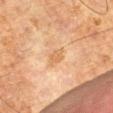Assessment: Part of a total-body skin-imaging series; this lesion was reviewed on a skin check and was not flagged for biopsy. Acquisition and patient details: Automated image analysis of the tile measured a lesion color around L≈51 a*≈17 b*≈33 in CIELAB, roughly 6 lightness units darker than nearby skin, and a lesion-to-skin contrast of about 5.5 (normalized; higher = more distinct). The analysis additionally found border irregularity of about 3 on a 0–10 scale, a color-variation rating of about 1/10, and a peripheral color-asymmetry measure near 0.5. From the left thigh. Approximately 2.5 mm at its widest. Imaged with cross-polarized lighting. A region of skin cropped from a whole-body photographic capture, roughly 15 mm wide. A male patient, roughly 80 years of age.